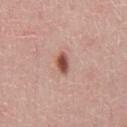Q: Was a biopsy performed?
A: imaged on a skin check; not biopsied
Q: Who is the patient?
A: male, roughly 45 years of age
Q: What kind of image is this?
A: ~15 mm crop, total-body skin-cancer survey
Q: Automated lesion metrics?
A: a footprint of about 4 mm² and a shape eccentricity near 0.8; a border-irregularity index near 3/10, a within-lesion color-variation index near 3.5/10, and radial color variation of about 1; a nevus-likeness score of about 100/100 and a lesion-detection confidence of about 100/100
Q: What is the anatomic site?
A: the chest
Q: How large is the lesion?
A: ≈3 mm
Q: How was the tile lit?
A: white-light